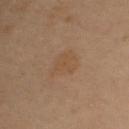This lesion was catalogued during total-body skin photography and was not selected for biopsy.
A lesion tile, about 15 mm wide, cut from a 3D total-body photograph.
Approximately 4 mm at its widest.
The lesion is on the upper back.
A female subject, aged 58 to 62.